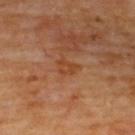biopsy_status: not biopsied; imaged during a skin examination
patient:
  sex: male
  age_approx: 60
image:
  source: total-body photography crop
  field_of_view_mm: 15
lighting: cross-polarized
site: upper back